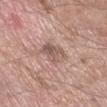Impression:
No biopsy was performed on this lesion — it was imaged during a full skin examination and was not determined to be concerning.
Background:
The patient is a male about 60 years old. A roughly 15 mm field-of-view crop from a total-body skin photograph. From the right forearm.